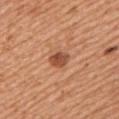biopsy_status: not biopsied; imaged during a skin examination
lesion_size:
  long_diameter_mm_approx: 2.5
patient:
  sex: male
  age_approx: 55
lighting: white-light
image:
  source: total-body photography crop
  field_of_view_mm: 15
site: left upper arm